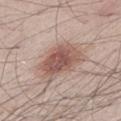Impression:
Recorded during total-body skin imaging; not selected for excision or biopsy.
Acquisition and patient details:
The recorded lesion diameter is about 6 mm. The subject is a male about 25 years old. Cropped from a whole-body photographic skin survey; the tile spans about 15 mm. Captured under white-light illumination. Automated image analysis of the tile measured a border-irregularity rating of about 2.5/10 and a color-variation rating of about 5/10. The analysis additionally found a classifier nevus-likeness of about 95/100 and a detector confidence of about 100 out of 100 that the crop contains a lesion. Located on the left lower leg.